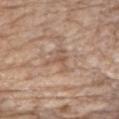The lesion was photographed on a routine skin check and not biopsied; there is no pathology result.